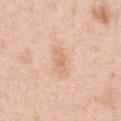Part of a total-body skin-imaging series; this lesion was reviewed on a skin check and was not flagged for biopsy.
Located on the front of the torso.
This image is a 15 mm lesion crop taken from a total-body photograph.
The patient is a male about 50 years old.
Captured under white-light illumination.
The recorded lesion diameter is about 3.5 mm.